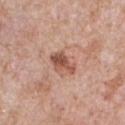The lesion was photographed on a routine skin check and not biopsied; there is no pathology result. Captured under white-light illumination. The subject is a male aged approximately 60. Located on the front of the torso. A 15 mm close-up extracted from a 3D total-body photography capture. About 3.5 mm across.